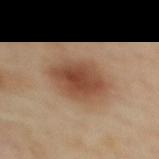About 6.5 mm across.
The lesion is on the upper back.
This image is a 15 mm lesion crop taken from a total-body photograph.
Automated tile analysis of the lesion measured a lesion area of about 18 mm², an eccentricity of roughly 0.8, and a shape-asymmetry score of about 0.15 (0 = symmetric). The software also gave border irregularity of about 1.5 on a 0–10 scale, a color-variation rating of about 4.5/10, and radial color variation of about 1.5. The analysis additionally found a lesion-detection confidence of about 100/100.
A female patient, aged 38–42.